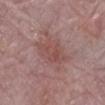Assessment:
Imaged during a routine full-body skin examination; the lesion was not biopsied and no histopathology is available.
Image and clinical context:
A lesion tile, about 15 mm wide, cut from a 3D total-body photograph. A female subject roughly 65 years of age. Approximately 4.5 mm at its widest. The lesion is on the leg. The tile uses white-light illumination.